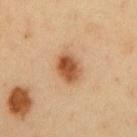<tbp_lesion>
<biopsy_status>not biopsied; imaged during a skin examination</biopsy_status>
<image>
  <source>total-body photography crop</source>
  <field_of_view_mm>15</field_of_view_mm>
</image>
<automated_metrics>
  <border_irregularity_0_10>1.0</border_irregularity_0_10>
  <color_variation_0_10>6.0</color_variation_0_10>
</automated_metrics>
<lighting>cross-polarized</lighting>
<site>chest</site>
<lesion_size>
  <long_diameter_mm_approx>3.5</long_diameter_mm_approx>
</lesion_size>
<patient>
  <sex>male</sex>
  <age_approx>50</age_approx>
</patient>
</tbp_lesion>